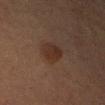biopsy_status: not biopsied; imaged during a skin examination
lesion_size:
  long_diameter_mm_approx: 3.0
image:
  source: total-body photography crop
  field_of_view_mm: 15
lighting: cross-polarized
automated_metrics:
  cielab_L: 23
  cielab_a: 14
  cielab_b: 20
  vs_skin_darker_L: 6.0
  vs_skin_contrast_norm: 7.0
  border_irregularity_0_10: 1.5
patient:
  sex: male
  age_approx: 75
site: right upper arm